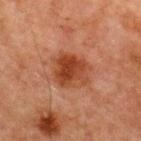<record>
<biopsy_status>not biopsied; imaged during a skin examination</biopsy_status>
<patient>
  <sex>male</sex>
  <age_approx>65</age_approx>
</patient>
<lighting>cross-polarized</lighting>
<image>
  <source>total-body photography crop</source>
  <field_of_view_mm>15</field_of_view_mm>
</image>
<automated_metrics>
  <cielab_L>35</cielab_L>
  <cielab_a>23</cielab_a>
  <cielab_b>30</cielab_b>
  <vs_skin_darker_L>9.0</vs_skin_darker_L>
  <vs_skin_contrast_norm>8.5</vs_skin_contrast_norm>
  <border_irregularity_0_10>2.0</border_irregularity_0_10>
  <color_variation_0_10>5.5</color_variation_0_10>
  <nevus_likeness_0_100>80</nevus_likeness_0_100>
</automated_metrics>
<site>chest</site>
<lesion_size>
  <long_diameter_mm_approx>5.0</long_diameter_mm_approx>
</lesion_size>
</record>